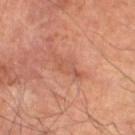– follow-up — imaged on a skin check; not biopsied